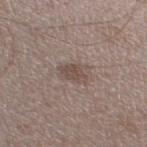Part of a total-body skin-imaging series; this lesion was reviewed on a skin check and was not flagged for biopsy. The subject is a male aged 48–52. Imaged with white-light lighting. The lesion's longest dimension is about 3 mm. A 15 mm close-up extracted from a 3D total-body photography capture. On the left thigh.